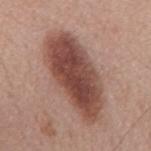| key | value |
|---|---|
| biopsy status | imaged on a skin check; not biopsied |
| subject | male, aged 68 to 72 |
| image source | ~15 mm tile from a whole-body skin photo |
| site | the mid back |
| tile lighting | white-light |
| diameter | ≈10 mm |